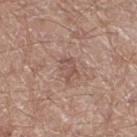Q: Is there a histopathology result?
A: catalogued during a skin exam; not biopsied
Q: Lesion size?
A: ≈3 mm
Q: Patient demographics?
A: male, in their mid-60s
Q: Automated lesion metrics?
A: border irregularity of about 5.5 on a 0–10 scale, a color-variation rating of about 0.5/10, and radial color variation of about 0
Q: What is the imaging modality?
A: ~15 mm tile from a whole-body skin photo
Q: Lesion location?
A: the right thigh